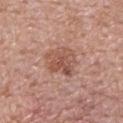Captured during whole-body skin photography for melanoma surveillance; the lesion was not biopsied.
A male patient, in their mid-50s.
Located on the upper back.
Cropped from a whole-body photographic skin survey; the tile spans about 15 mm.